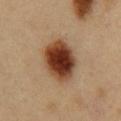biopsy_status: not biopsied; imaged during a skin examination
lesion_size:
  long_diameter_mm_approx: 6.0
site: chest
image:
  source: total-body photography crop
  field_of_view_mm: 15
patient:
  sex: male
  age_approx: 50
lighting: cross-polarized